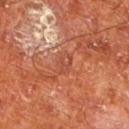| feature | finding |
|---|---|
| workup | total-body-photography surveillance lesion; no biopsy |
| anatomic site | the left lower leg |
| imaging modality | 15 mm crop, total-body photography |
| lesion diameter | ≈3 mm |
| tile lighting | cross-polarized |
| patient | male, approximately 65 years of age |